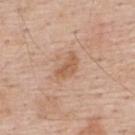Notes:
– follow-up · no biopsy performed (imaged during a skin exam)
– acquisition · ~15 mm tile from a whole-body skin photo
– patient · male, in their 60s
– illumination · white-light illumination
– location · the back
– automated lesion analysis · an area of roughly 5 mm², a shape eccentricity near 0.85, and a shape-asymmetry score of about 0.4 (0 = symmetric); border irregularity of about 3.5 on a 0–10 scale, internal color variation of about 2 on a 0–10 scale, and a peripheral color-asymmetry measure near 1; a nevus-likeness score of about 10/100 and a lesion-detection confidence of about 100/100